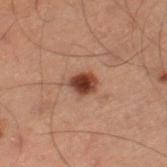Captured during whole-body skin photography for melanoma surveillance; the lesion was not biopsied.
The lesion is located on the left thigh.
Automated image analysis of the tile measured a lesion area of about 5 mm² and a symmetry-axis asymmetry near 0.15. And it measured a lesion color around L≈30 a*≈19 b*≈23 in CIELAB, a lesion–skin lightness drop of about 13, and a normalized border contrast of about 12.
A 15 mm crop from a total-body photograph taken for skin-cancer surveillance.
Measured at roughly 3 mm in maximum diameter.
The patient is a male aged 58 to 62.
The tile uses cross-polarized illumination.